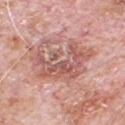Case summary:
* workup: no biopsy performed (imaged during a skin exam)
* patient: male, in their 80s
* imaging modality: ~15 mm crop, total-body skin-cancer survey
* body site: the chest